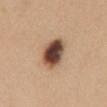Captured during whole-body skin photography for melanoma surveillance; the lesion was not biopsied.
The lesion is on the mid back.
A 15 mm close-up tile from a total-body photography series done for melanoma screening.
The recorded lesion diameter is about 5 mm.
The tile uses white-light illumination.
A female patient roughly 30 years of age.
The lesion-visualizer software estimated a lesion area of about 12 mm², a shape eccentricity near 0.85, and two-axis asymmetry of about 0.15. The software also gave roughly 21 lightness units darker than nearby skin and a normalized border contrast of about 14. And it measured a border-irregularity index near 2/10, internal color variation of about 9.5 on a 0–10 scale, and peripheral color asymmetry of about 3. The software also gave a classifier nevus-likeness of about 95/100 and a detector confidence of about 100 out of 100 that the crop contains a lesion.